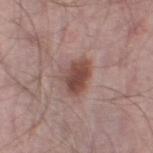automated lesion analysis: a peripheral color-asymmetry measure near 1.5; a nevus-likeness score of about 90/100 and a lesion-detection confidence of about 100/100 | acquisition: ~15 mm crop, total-body skin-cancer survey | subject: male, roughly 65 years of age | lesion diameter: ~4 mm (longest diameter) | anatomic site: the leg.